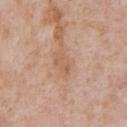Case summary:
- notes — total-body-photography surveillance lesion; no biopsy
- tile lighting — white-light
- location — the chest
- image source — total-body-photography crop, ~15 mm field of view
- lesion diameter — ≈3 mm
- subject — male, in their mid- to late 60s